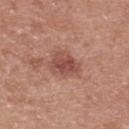No biopsy was performed on this lesion — it was imaged during a full skin examination and was not determined to be concerning. From the upper back. Automated image analysis of the tile measured a footprint of about 8 mm², a shape eccentricity near 0.6, and a symmetry-axis asymmetry near 0.25. And it measured a lesion color around L≈49 a*≈25 b*≈26 in CIELAB, about 10 CIELAB-L* units darker than the surrounding skin, and a lesion-to-skin contrast of about 7.5 (normalized; higher = more distinct). And it measured a lesion-detection confidence of about 100/100. This image is a 15 mm lesion crop taken from a total-body photograph. The lesion's longest dimension is about 3.5 mm. A female subject, aged 38–42. Imaged with white-light lighting.